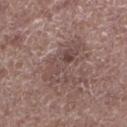workup = imaged on a skin check; not biopsied
tile lighting = white-light
location = the leg
patient = male, in their 70s
lesion size = about 7 mm
image = ~15 mm crop, total-body skin-cancer survey